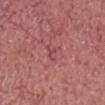notes: catalogued during a skin exam; not biopsied
size: ~2.5 mm (longest diameter)
acquisition: ~15 mm crop, total-body skin-cancer survey
site: the head or neck
patient: male, roughly 75 years of age
automated lesion analysis: an average lesion color of about L≈48 a*≈31 b*≈20 (CIELAB), a lesion–skin lightness drop of about 6, and a lesion-to-skin contrast of about 4.5 (normalized; higher = more distinct); a peripheral color-asymmetry measure near 0; a classifier nevus-likeness of about 0/100 and lesion-presence confidence of about 60/100
lighting: white-light illumination